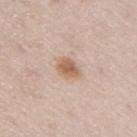Q: Was a biopsy performed?
A: no biopsy performed (imaged during a skin exam)
Q: Who is the patient?
A: female, aged approximately 50
Q: What kind of image is this?
A: ~15 mm tile from a whole-body skin photo
Q: Illumination type?
A: white-light illumination
Q: Lesion size?
A: ~3 mm (longest diameter)
Q: Where on the body is the lesion?
A: the right upper arm